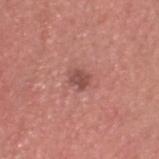follow-up: catalogued during a skin exam; not biopsied | automated metrics: a footprint of about 3 mm² and a shape-asymmetry score of about 0.3 (0 = symmetric); border irregularity of about 2.5 on a 0–10 scale and internal color variation of about 2 on a 0–10 scale; a nevus-likeness score of about 30/100 and a lesion-detection confidence of about 100/100 | lesion diameter: about 2 mm | location: the head or neck | patient: male, roughly 40 years of age | imaging modality: total-body-photography crop, ~15 mm field of view | lighting: white-light illumination.